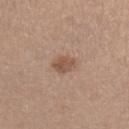The lesion was tiled from a total-body skin photograph and was not biopsied. This image is a 15 mm lesion crop taken from a total-body photograph. Located on the right upper arm. A female subject aged approximately 65.